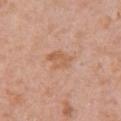Recorded during total-body skin imaging; not selected for excision or biopsy. The lesion-visualizer software estimated an outline eccentricity of about 0.75 (0 = round, 1 = elongated) and two-axis asymmetry of about 0.3. It also reported a lesion color around L≈59 a*≈22 b*≈34 in CIELAB, roughly 7 lightness units darker than nearby skin, and a normalized border contrast of about 6. The analysis additionally found a border-irregularity index near 3/10 and radial color variation of about 0.5. The analysis additionally found an automated nevus-likeness rating near 0 out of 100 and a lesion-detection confidence of about 100/100. The tile uses white-light illumination. The lesion's longest dimension is about 3.5 mm. A lesion tile, about 15 mm wide, cut from a 3D total-body photograph. From the chest. A female subject aged 38–42.